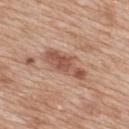biopsy status = total-body-photography surveillance lesion; no biopsy
subject = female, aged 38 to 42
diameter = ≈6 mm
illumination = white-light illumination
location = the back
imaging modality = ~15 mm crop, total-body skin-cancer survey